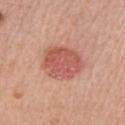No biopsy was performed on this lesion — it was imaged during a full skin examination and was not determined to be concerning. A female patient, roughly 60 years of age. Imaged with white-light lighting. A close-up tile cropped from a whole-body skin photograph, about 15 mm across. Longest diameter approximately 4.5 mm. Located on the arm.